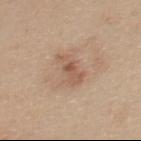Impression:
The lesion was tiled from a total-body skin photograph and was not biopsied.
Context:
Automated tile analysis of the lesion measured an area of roughly 7 mm², an outline eccentricity of about 0.9 (0 = round, 1 = elongated), and a symmetry-axis asymmetry near 0.35. It also reported a lesion-detection confidence of about 100/100. On the upper back. A close-up tile cropped from a whole-body skin photograph, about 15 mm across. The patient is a female in their mid-20s. About 5 mm across.